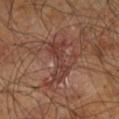Q: Is there a histopathology result?
A: total-body-photography surveillance lesion; no biopsy